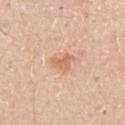workup: catalogued during a skin exam; not biopsied | automated metrics: a within-lesion color-variation index near 2.5/10; an automated nevus-likeness rating near 35 out of 100 and a detector confidence of about 100 out of 100 that the crop contains a lesion | patient: male, approximately 60 years of age | image: total-body-photography crop, ~15 mm field of view | body site: the right upper arm.